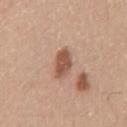Clinical impression: Imaged during a routine full-body skin examination; the lesion was not biopsied and no histopathology is available. Clinical summary: The lesion is located on the mid back. A region of skin cropped from a whole-body photographic capture, roughly 15 mm wide. A male subject, aged around 30.